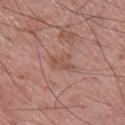Q: Was a biopsy performed?
A: no biopsy performed (imaged during a skin exam)
Q: Lesion size?
A: ≈3.5 mm
Q: What kind of image is this?
A: 15 mm crop, total-body photography
Q: Who is the patient?
A: male, aged 68 to 72
Q: What is the anatomic site?
A: the leg